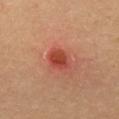A female subject, roughly 35 years of age.
A region of skin cropped from a whole-body photographic capture, roughly 15 mm wide.
The recorded lesion diameter is about 3 mm.
Imaged with cross-polarized lighting.
An algorithmic analysis of the crop reported a mean CIELAB color near L≈46 a*≈33 b*≈33, roughly 11 lightness units darker than nearby skin, and a lesion-to-skin contrast of about 8 (normalized; higher = more distinct). The analysis additionally found border irregularity of about 1 on a 0–10 scale, internal color variation of about 4 on a 0–10 scale, and a peripheral color-asymmetry measure near 1.5.
The lesion is located on the leg.